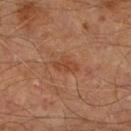No biopsy was performed on this lesion — it was imaged during a full skin examination and was not determined to be concerning. Cropped from a whole-body photographic skin survey; the tile spans about 15 mm. A male patient, approximately 65 years of age. On the right lower leg.